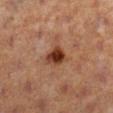No biopsy was performed on this lesion — it was imaged during a full skin examination and was not determined to be concerning. A female subject, aged around 40. A close-up tile cropped from a whole-body skin photograph, about 15 mm across. On the right lower leg.